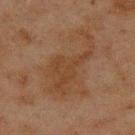{"biopsy_status": "not biopsied; imaged during a skin examination"}